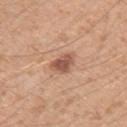Captured during whole-body skin photography for melanoma surveillance; the lesion was not biopsied. Measured at roughly 5 mm in maximum diameter. Captured under white-light illumination. On the left upper arm. The patient is a male roughly 30 years of age. A lesion tile, about 15 mm wide, cut from a 3D total-body photograph. Automated tile analysis of the lesion measured a footprint of about 13 mm², an eccentricity of roughly 0.6, and a symmetry-axis asymmetry near 0.35. The software also gave a lesion-to-skin contrast of about 5.5 (normalized; higher = more distinct). The analysis additionally found border irregularity of about 4.5 on a 0–10 scale and a peripheral color-asymmetry measure near 1.5. The analysis additionally found a nevus-likeness score of about 85/100 and a detector confidence of about 100 out of 100 that the crop contains a lesion.